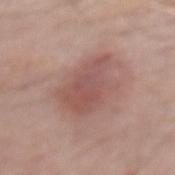Q: Was this lesion biopsied?
A: no biopsy performed (imaged during a skin exam)
Q: What kind of image is this?
A: ~15 mm tile from a whole-body skin photo
Q: How large is the lesion?
A: ≈6 mm
Q: Who is the patient?
A: male, aged 68–72
Q: What lighting was used for the tile?
A: white-light illumination
Q: What is the anatomic site?
A: the back
Q: What did automated image analysis measure?
A: a footprint of about 17 mm²; a lesion color around L≈53 a*≈22 b*≈23 in CIELAB, roughly 9 lightness units darker than nearby skin, and a lesion-to-skin contrast of about 6.5 (normalized; higher = more distinct); a within-lesion color-variation index near 3.5/10 and peripheral color asymmetry of about 1; a nevus-likeness score of about 40/100